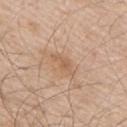Imaged during a routine full-body skin examination; the lesion was not biopsied and no histopathology is available.
The subject is a male approximately 50 years of age.
About 3.5 mm across.
From the right upper arm.
A 15 mm crop from a total-body photograph taken for skin-cancer surveillance.